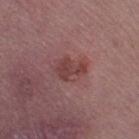The lesion was photographed on a routine skin check and not biopsied; there is no pathology result.
A lesion tile, about 15 mm wide, cut from a 3D total-body photograph.
The subject is a female roughly 55 years of age.
The tile uses white-light illumination.
The total-body-photography lesion software estimated border irregularity of about 5 on a 0–10 scale and internal color variation of about 2 on a 0–10 scale. And it measured a classifier nevus-likeness of about 10/100 and a lesion-detection confidence of about 100/100.
The lesion's longest dimension is about 3.5 mm.
From the left thigh.